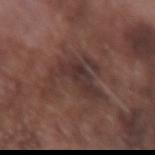{"biopsy_status": "not biopsied; imaged during a skin examination", "lesion_size": {"long_diameter_mm_approx": 7.0}, "lighting": "white-light", "patient": {"sex": "male", "age_approx": 75}, "site": "right forearm", "image": {"source": "total-body photography crop", "field_of_view_mm": 15}}